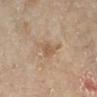Captured during whole-body skin photography for melanoma surveillance; the lesion was not biopsied.
A female subject, roughly 80 years of age.
The total-body-photography lesion software estimated a mean CIELAB color near L≈47 a*≈14 b*≈27 and a normalized lesion–skin contrast near 6.
Located on the right lower leg.
A close-up tile cropped from a whole-body skin photograph, about 15 mm across.